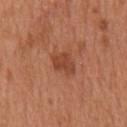This lesion was catalogued during total-body skin photography and was not selected for biopsy.
The patient is a male aged around 65.
An algorithmic analysis of the crop reported a lesion area of about 5 mm², an outline eccentricity of about 0.75 (0 = round, 1 = elongated), and a symmetry-axis asymmetry near 0.3. It also reported an average lesion color of about L≈45 a*≈27 b*≈33 (CIELAB) and a normalized border contrast of about 7. And it measured border irregularity of about 3 on a 0–10 scale, a within-lesion color-variation index near 2/10, and peripheral color asymmetry of about 0.5.
This is a white-light tile.
This image is a 15 mm lesion crop taken from a total-body photograph.
Located on the mid back.
Approximately 3.5 mm at its widest.